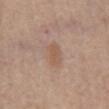Notes:
* biopsy status: total-body-photography surveillance lesion; no biopsy
* site: the chest
* lighting: white-light illumination
* subject: male, about 65 years old
* image: 15 mm crop, total-body photography
* size: ≈3 mm